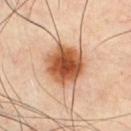This lesion was catalogued during total-body skin photography and was not selected for biopsy.
A male subject, aged approximately 50.
The total-body-photography lesion software estimated about 19 CIELAB-L* units darker than the surrounding skin and a normalized lesion–skin contrast near 12.5. And it measured an automated nevus-likeness rating near 100 out of 100.
Longest diameter approximately 5 mm.
On the front of the torso.
A roughly 15 mm field-of-view crop from a total-body skin photograph.
Captured under cross-polarized illumination.